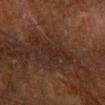follow-up: imaged on a skin check; not biopsied
image: total-body-photography crop, ~15 mm field of view
lesion size: about 3 mm
subject: male, in their 60s
site: the right forearm
tile lighting: cross-polarized illumination